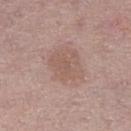Clinical impression:
The lesion was tiled from a total-body skin photograph and was not biopsied.
Background:
The patient is a female about 40 years old. The lesion is located on the left thigh. A close-up tile cropped from a whole-body skin photograph, about 15 mm across. Captured under white-light illumination.